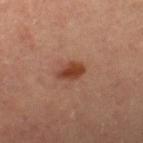No biopsy was performed on this lesion — it was imaged during a full skin examination and was not determined to be concerning. Approximately 3 mm at its widest. A female patient aged around 45. A close-up tile cropped from a whole-body skin photograph, about 15 mm across. On the right lower leg.